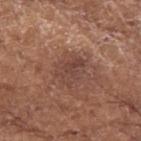workup: imaged on a skin check; not biopsied | image-analysis metrics: a border-irregularity index near 3.5/10, internal color variation of about 3 on a 0–10 scale, and radial color variation of about 1 | patient: female, aged 73 to 77 | image source: total-body-photography crop, ~15 mm field of view | illumination: white-light | diameter: about 3.5 mm | body site: the arm.